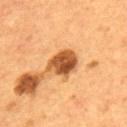Notes:
- notes: no biopsy performed (imaged during a skin exam)